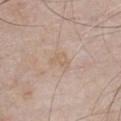biopsy status: catalogued during a skin exam; not biopsied
imaging modality: ~15 mm tile from a whole-body skin photo
patient: male, about 55 years old
anatomic site: the chest